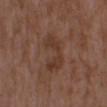<case>
<biopsy_status>not biopsied; imaged during a skin examination</biopsy_status>
<patient>
  <sex>female</sex>
  <age_approx>30</age_approx>
</patient>
<automated_metrics>
  <vs_skin_darker_L>6.0</vs_skin_darker_L>
  <vs_skin_contrast_norm>6.0</vs_skin_contrast_norm>
  <nevus_likeness_0_100>0</nevus_likeness_0_100>
  <lesion_detection_confidence_0_100>100</lesion_detection_confidence_0_100>
</automated_metrics>
<lighting>white-light</lighting>
<lesion_size>
  <long_diameter_mm_approx>5.0</long_diameter_mm_approx>
</lesion_size>
<site>upper back</site>
<image>
  <source>total-body photography crop</source>
  <field_of_view_mm>15</field_of_view_mm>
</image>
</case>